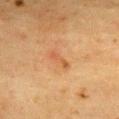| field | value |
|---|---|
| notes | total-body-photography surveillance lesion; no biopsy |
| patient | female, aged 53 to 57 |
| automated metrics | a within-lesion color-variation index near 0/10 and radial color variation of about 0; an automated nevus-likeness rating near 0 out of 100 and lesion-presence confidence of about 100/100 |
| size | ~3 mm (longest diameter) |
| location | the chest |
| image | 15 mm crop, total-body photography |